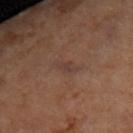The lesion is on the upper back.
A region of skin cropped from a whole-body photographic capture, roughly 15 mm wide.
The patient is a female roughly 45 years of age.